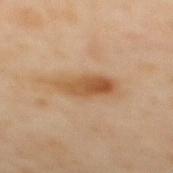Notes:
- follow-up — no biopsy performed (imaged during a skin exam)
- body site — the mid back
- subject — male, in their mid-60s
- image — ~15 mm crop, total-body skin-cancer survey